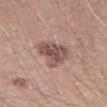Impression: No biopsy was performed on this lesion — it was imaged during a full skin examination and was not determined to be concerning. Background: The patient is a male about 30 years old. A close-up tile cropped from a whole-body skin photograph, about 15 mm across.